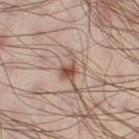Clinical impression: Imaged during a routine full-body skin examination; the lesion was not biopsied and no histopathology is available. Image and clinical context: About 3.5 mm across. A roughly 15 mm field-of-view crop from a total-body skin photograph. The patient is a male in their 40s. On the right thigh. The lesion-visualizer software estimated a lesion area of about 4 mm² and two-axis asymmetry of about 0.5. And it measured a lesion color around L≈50 a*≈19 b*≈27 in CIELAB.